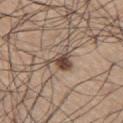The lesion was tiled from a total-body skin photograph and was not biopsied.
On the right thigh.
Imaged with white-light lighting.
The lesion's longest dimension is about 3.5 mm.
A 15 mm close-up extracted from a 3D total-body photography capture.
The patient is a male aged around 65.